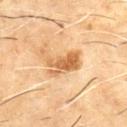Image and clinical context: This is a cross-polarized tile. Cropped from a whole-body photographic skin survey; the tile spans about 15 mm. A male subject about 60 years old. Longest diameter approximately 4.5 mm. From the chest.